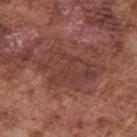notes: catalogued during a skin exam; not biopsied | imaging modality: 15 mm crop, total-body photography | patient: male, aged approximately 75 | location: the right upper arm.